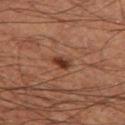No biopsy was performed on this lesion — it was imaged during a full skin examination and was not determined to be concerning.